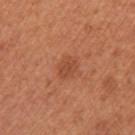Q: Is there a histopathology result?
A: total-body-photography surveillance lesion; no biopsy
Q: What are the patient's age and sex?
A: female, approximately 65 years of age
Q: What is the imaging modality?
A: total-body-photography crop, ~15 mm field of view
Q: Lesion location?
A: the arm
Q: How large is the lesion?
A: ~2.5 mm (longest diameter)